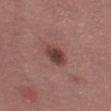| feature | finding |
|---|---|
| follow-up | imaged on a skin check; not biopsied |
| patient | female, aged around 55 |
| diameter | about 3.5 mm |
| location | the leg |
| acquisition | 15 mm crop, total-body photography |